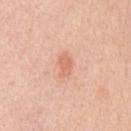{
  "site": "front of the torso",
  "patient": {
    "sex": "male",
    "age_approx": 50
  },
  "image": {
    "source": "total-body photography crop",
    "field_of_view_mm": 15
  }
}